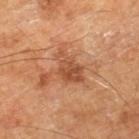<case>
  <patient>
    <sex>male</sex>
    <age_approx>65</age_approx>
  </patient>
  <site>leg</site>
  <image>
    <source>total-body photography crop</source>
    <field_of_view_mm>15</field_of_view_mm>
  </image>
  <lesion_size>
    <long_diameter_mm_approx>4.5</long_diameter_mm_approx>
  </lesion_size>
</case>